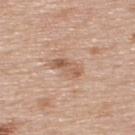No biopsy was performed on this lesion — it was imaged during a full skin examination and was not determined to be concerning.